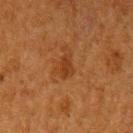<case>
  <biopsy_status>not biopsied; imaged during a skin examination</biopsy_status>
  <site>left upper arm</site>
  <image>
    <source>total-body photography crop</source>
    <field_of_view_mm>15</field_of_view_mm>
  </image>
  <patient>
    <sex>female</sex>
    <age_approx>50</age_approx>
  </patient>
</case>